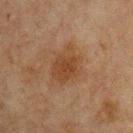Part of a total-body skin-imaging series; this lesion was reviewed on a skin check and was not flagged for biopsy. The lesion's longest dimension is about 4.5 mm. This image is a 15 mm lesion crop taken from a total-body photograph. The lesion is on the back. This is a cross-polarized tile. Automated tile analysis of the lesion measured a footprint of about 11 mm², an eccentricity of roughly 0.65, and a symmetry-axis asymmetry near 0.25. And it measured a lesion color around L≈35 a*≈17 b*≈28 in CIELAB and a lesion–skin lightness drop of about 7. It also reported a border-irregularity index near 3/10, a within-lesion color-variation index near 2.5/10, and a peripheral color-asymmetry measure near 1. It also reported a detector confidence of about 100 out of 100 that the crop contains a lesion. A male patient approximately 65 years of age.